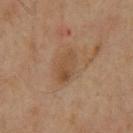Clinical impression: Recorded during total-body skin imaging; not selected for excision or biopsy. Clinical summary: The lesion's longest dimension is about 4.5 mm. On the chest. Cropped from a total-body skin-imaging series; the visible field is about 15 mm. A male patient, aged 58–62.